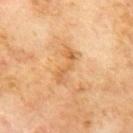| field | value |
|---|---|
| follow-up | total-body-photography surveillance lesion; no biopsy |
| TBP lesion metrics | an area of roughly 5 mm² and an outline eccentricity of about 0.95 (0 = round, 1 = elongated); border irregularity of about 6.5 on a 0–10 scale and peripheral color asymmetry of about 0.5; a nevus-likeness score of about 0/100 |
| acquisition | ~15 mm tile from a whole-body skin photo |
| lighting | cross-polarized illumination |
| body site | the mid back |
| subject | male, approximately 70 years of age |
| diameter | about 4.5 mm |